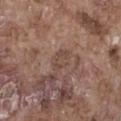notes: catalogued during a skin exam; not biopsied
location: the abdomen
automated metrics: a border-irregularity rating of about 4/10 and a within-lesion color-variation index near 1.5/10
subject: male, aged around 75
imaging modality: total-body-photography crop, ~15 mm field of view
lesion diameter: ≈2.5 mm